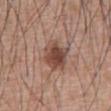Findings:
• notes: catalogued during a skin exam; not biopsied
• subject: male, aged 58 to 62
• tile lighting: white-light
• image source: ~15 mm crop, total-body skin-cancer survey
• diameter: ≈4 mm
• site: the abdomen